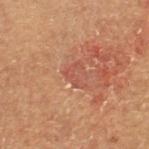Clinical impression: No biopsy was performed on this lesion — it was imaged during a full skin examination and was not determined to be concerning. Background: A 15 mm close-up tile from a total-body photography series done for melanoma screening. A male patient aged 63–67. Automated tile analysis of the lesion measured a border-irregularity rating of about 8/10, internal color variation of about 0 on a 0–10 scale, and radial color variation of about 0. Captured under cross-polarized illumination. Located on the right thigh.